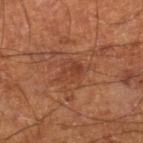This lesion was catalogued during total-body skin photography and was not selected for biopsy. The tile uses cross-polarized illumination. The subject is a male about 60 years old. The lesion is located on the right lower leg. The lesion's longest dimension is about 3 mm. This image is a 15 mm lesion crop taken from a total-body photograph.